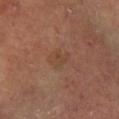biopsy_status: not biopsied; imaged during a skin examination
lighting: cross-polarized
site: left lower leg
patient:
  sex: male
  age_approx: 65
lesion_size:
  long_diameter_mm_approx: 2.5
image:
  source: total-body photography crop
  field_of_view_mm: 15